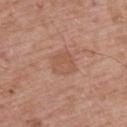Q: Was this lesion biopsied?
A: imaged on a skin check; not biopsied
Q: Lesion location?
A: the upper back
Q: How was the tile lit?
A: white-light illumination
Q: What is the imaging modality?
A: total-body-photography crop, ~15 mm field of view
Q: What did automated image analysis measure?
A: an average lesion color of about L≈54 a*≈22 b*≈30 (CIELAB), roughly 6 lightness units darker than nearby skin, and a normalized lesion–skin contrast near 5; a border-irregularity rating of about 2/10, a color-variation rating of about 2/10, and radial color variation of about 0.5; a nevus-likeness score of about 0/100 and a lesion-detection confidence of about 100/100
Q: Who is the patient?
A: male, aged approximately 65
Q: What is the lesion's diameter?
A: about 3 mm